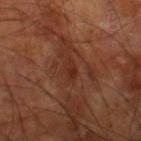Q: Was a biopsy performed?
A: no biopsy performed (imaged during a skin exam)
Q: What did automated image analysis measure?
A: a mean CIELAB color near L≈29 a*≈23 b*≈28 and a lesion-to-skin contrast of about 6 (normalized; higher = more distinct); a border-irregularity index near 2.5/10 and a peripheral color-asymmetry measure near 1
Q: Illumination type?
A: cross-polarized illumination
Q: What are the patient's age and sex?
A: male, approximately 60 years of age
Q: What kind of image is this?
A: 15 mm crop, total-body photography
Q: What is the anatomic site?
A: the right lower leg
Q: What is the lesion's diameter?
A: ~2.5 mm (longest diameter)